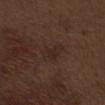Imaged during a routine full-body skin examination; the lesion was not biopsied and no histopathology is available. Located on the abdomen. A 15 mm close-up extracted from a 3D total-body photography capture. Longest diameter approximately 3.5 mm. A male subject aged approximately 70. Automated image analysis of the tile measured an area of roughly 5.5 mm² and a shape-asymmetry score of about 0.35 (0 = symmetric). The software also gave a mean CIELAB color near L≈25 a*≈15 b*≈20, about 4 CIELAB-L* units darker than the surrounding skin, and a lesion-to-skin contrast of about 5 (normalized; higher = more distinct). The analysis additionally found a classifier nevus-likeness of about 15/100. This is a white-light tile.